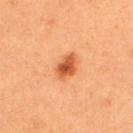biopsy status: catalogued during a skin exam; not biopsied | subject: male, about 35 years old | acquisition: ~15 mm tile from a whole-body skin photo | lighting: cross-polarized illumination | automated metrics: a footprint of about 5.5 mm²; roughly 14 lightness units darker than nearby skin and a normalized border contrast of about 9; border irregularity of about 2 on a 0–10 scale, a color-variation rating of about 4.5/10, and radial color variation of about 1.5 | lesion size: ≈3.5 mm | location: the back.